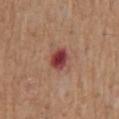Impression:
No biopsy was performed on this lesion — it was imaged during a full skin examination and was not determined to be concerning.
Image and clinical context:
The recorded lesion diameter is about 3 mm. The lesion is on the back. The tile uses white-light illumination. A close-up tile cropped from a whole-body skin photograph, about 15 mm across. A male subject in their 70s.